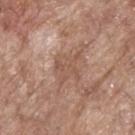The lesion is on the upper back. Cropped from a whole-body photographic skin survey; the tile spans about 15 mm. The subject is a male approximately 70 years of age.